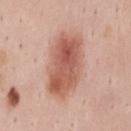Imaged during a routine full-body skin examination; the lesion was not biopsied and no histopathology is available. The lesion is on the mid back. The lesion's longest dimension is about 7.5 mm. A male subject, aged around 35. This image is a 15 mm lesion crop taken from a total-body photograph. The total-body-photography lesion software estimated about 13 CIELAB-L* units darker than the surrounding skin and a lesion-to-skin contrast of about 9 (normalized; higher = more distinct).